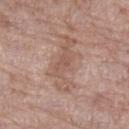Q: Was a biopsy performed?
A: catalogued during a skin exam; not biopsied
Q: What is the lesion's diameter?
A: ≈7 mm
Q: Where on the body is the lesion?
A: the left lower leg
Q: Patient demographics?
A: female, roughly 85 years of age or older
Q: Illumination type?
A: white-light illumination
Q: How was this image acquired?
A: total-body-photography crop, ~15 mm field of view
Q: What did automated image analysis measure?
A: an eccentricity of roughly 0.9 and two-axis asymmetry of about 0.4; a border-irregularity index near 7/10, a within-lesion color-variation index near 3/10, and peripheral color asymmetry of about 1; a lesion-detection confidence of about 85/100